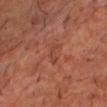No biopsy was performed on this lesion — it was imaged during a full skin examination and was not determined to be concerning. Approximately 2.5 mm at its widest. The tile uses cross-polarized illumination. A male patient, aged 53–57. A 15 mm close-up tile from a total-body photography series done for melanoma screening. On the chest. Automated image analysis of the tile measured an area of roughly 2.5 mm², an outline eccentricity of about 0.9 (0 = round, 1 = elongated), and a shape-asymmetry score of about 0.35 (0 = symmetric). And it measured a border-irregularity rating of about 4/10, internal color variation of about 0 on a 0–10 scale, and radial color variation of about 0.